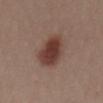Assessment:
Part of a total-body skin-imaging series; this lesion was reviewed on a skin check and was not flagged for biopsy.
Background:
A female subject about 30 years old. On the abdomen. Longest diameter approximately 5 mm. This is a white-light tile. A lesion tile, about 15 mm wide, cut from a 3D total-body photograph.